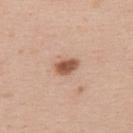This lesion was catalogued during total-body skin photography and was not selected for biopsy.
This is a white-light tile.
The lesion's longest dimension is about 3 mm.
A female subject, approximately 35 years of age.
Located on the upper back.
A 15 mm crop from a total-body photograph taken for skin-cancer surveillance.